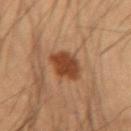location: the left forearm
diameter: ~3.5 mm (longest diameter)
tile lighting: cross-polarized illumination
image source: total-body-photography crop, ~15 mm field of view
patient: male, about 35 years old